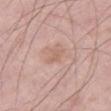| feature | finding |
|---|---|
| follow-up | catalogued during a skin exam; not biopsied |
| site | the left thigh |
| patient | male, in their mid- to late 50s |
| acquisition | ~15 mm crop, total-body skin-cancer survey |